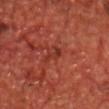subject: male, aged 68 to 72 | image source: ~15 mm tile from a whole-body skin photo | tile lighting: cross-polarized illumination | diameter: about 2.5 mm | anatomic site: the chest | image-analysis metrics: a footprint of about 2.5 mm², a shape eccentricity near 0.9, and two-axis asymmetry of about 0.4.